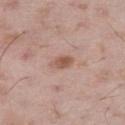biopsy status = imaged on a skin check; not biopsied | acquisition = 15 mm crop, total-body photography | patient = male, approximately 50 years of age | anatomic site = the right thigh.